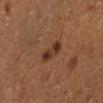follow-up: no biopsy performed (imaged during a skin exam) | automated lesion analysis: an area of roughly 5 mm² and a symmetry-axis asymmetry near 0.2; an average lesion color of about L≈27 a*≈16 b*≈23 (CIELAB), a lesion–skin lightness drop of about 8, and a normalized lesion–skin contrast near 9; a border-irregularity rating of about 2/10, internal color variation of about 3 on a 0–10 scale, and peripheral color asymmetry of about 1; a detector confidence of about 100 out of 100 that the crop contains a lesion | acquisition: 15 mm crop, total-body photography | body site: the leg | tile lighting: cross-polarized | patient: female, aged approximately 50 | lesion size: ~3 mm (longest diameter).